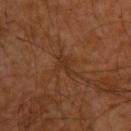Clinical impression: Captured during whole-body skin photography for melanoma surveillance; the lesion was not biopsied. Acquisition and patient details: The tile uses cross-polarized illumination. Located on the upper back. The patient is a male aged 28 to 32. Longest diameter approximately 3 mm. A 15 mm close-up tile from a total-body photography series done for melanoma screening. Automated image analysis of the tile measured a lesion color around L≈32 a*≈20 b*≈30 in CIELAB. It also reported border irregularity of about 6 on a 0–10 scale, a within-lesion color-variation index near 1/10, and a peripheral color-asymmetry measure near 0.5.